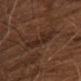Q: Was a biopsy performed?
A: imaged on a skin check; not biopsied
Q: What is the imaging modality?
A: 15 mm crop, total-body photography
Q: Patient demographics?
A: male, roughly 65 years of age